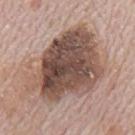Impression: No biopsy was performed on this lesion — it was imaged during a full skin examination and was not determined to be concerning. Clinical summary: The lesion's longest dimension is about 10 mm. This image is a 15 mm lesion crop taken from a total-body photograph. The subject is a male roughly 70 years of age. The tile uses white-light illumination. Located on the mid back.